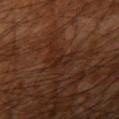{
  "biopsy_status": "not biopsied; imaged during a skin examination",
  "patient": {
    "sex": "male",
    "age_approx": 60
  },
  "lighting": "cross-polarized",
  "image": {
    "source": "total-body photography crop",
    "field_of_view_mm": 15
  },
  "site": "right upper arm",
  "lesion_size": {
    "long_diameter_mm_approx": 3.5
  }
}